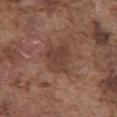Imaged during a routine full-body skin examination; the lesion was not biopsied and no histopathology is available.
About 4 mm across.
On the abdomen.
A lesion tile, about 15 mm wide, cut from a 3D total-body photograph.
This is a white-light tile.
A male subject, aged approximately 75.